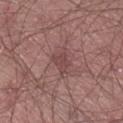workup: imaged on a skin check; not biopsied
tile lighting: white-light
imaging modality: total-body-photography crop, ~15 mm field of view
site: the lower back
subject: male, in their mid- to late 60s
automated metrics: a mean CIELAB color near L≈45 a*≈21 b*≈19, roughly 7 lightness units darker than nearby skin, and a lesion-to-skin contrast of about 6 (normalized; higher = more distinct); a within-lesion color-variation index near 2/10 and a peripheral color-asymmetry measure near 1; an automated nevus-likeness rating near 0 out of 100 and a detector confidence of about 90 out of 100 that the crop contains a lesion
lesion diameter: about 3 mm